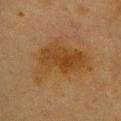workup: total-body-photography surveillance lesion; no biopsy
image source: ~15 mm crop, total-body skin-cancer survey
tile lighting: cross-polarized illumination
patient: female, about 40 years old
site: the upper back
automated metrics: a border-irregularity rating of about 5.5/10, internal color variation of about 4 on a 0–10 scale, and radial color variation of about 1.5